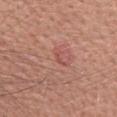{
  "biopsy_status": "not biopsied; imaged during a skin examination",
  "patient": {
    "sex": "female",
    "age_approx": 60
  },
  "image": {
    "source": "total-body photography crop",
    "field_of_view_mm": 15
  },
  "lesion_size": {
    "long_diameter_mm_approx": 1.5
  },
  "automated_metrics": {
    "area_mm2_approx": 1.0,
    "shape_asymmetry": 0.45,
    "border_irregularity_0_10": 4.5,
    "color_variation_0_10": 0.0,
    "peripheral_color_asymmetry": 0.0,
    "nevus_likeness_0_100": 45,
    "lesion_detection_confidence_0_100": 95
  },
  "lighting": "white-light",
  "site": "upper back"
}